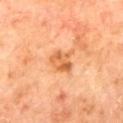Captured under cross-polarized illumination.
From the mid back.
The recorded lesion diameter is about 3 mm.
A close-up tile cropped from a whole-body skin photograph, about 15 mm across.
The lesion-visualizer software estimated a nevus-likeness score of about 0/100 and lesion-presence confidence of about 100/100.
The subject is a male aged around 70.